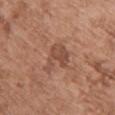follow-up = catalogued during a skin exam; not biopsied | location = the back | subject = female, in their mid- to late 70s | acquisition = total-body-photography crop, ~15 mm field of view.